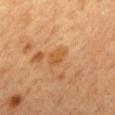Case summary:
- workup — total-body-photography surveillance lesion; no biopsy
- tile lighting — cross-polarized illumination
- anatomic site — the mid back
- subject — female, aged around 60
- lesion size — ~2.5 mm (longest diameter)
- acquisition — 15 mm crop, total-body photography